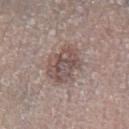Part of a total-body skin-imaging series; this lesion was reviewed on a skin check and was not flagged for biopsy. Located on the left lower leg. A 15 mm crop from a total-body photograph taken for skin-cancer surveillance. A male subject, roughly 55 years of age. Automated tile analysis of the lesion measured an outline eccentricity of about 0.75 (0 = round, 1 = elongated). The software also gave a lesion color around L≈50 a*≈15 b*≈20 in CIELAB and a lesion–skin lightness drop of about 10. And it measured a border-irregularity rating of about 2.5/10, a within-lesion color-variation index near 5.5/10, and peripheral color asymmetry of about 2. The software also gave lesion-presence confidence of about 85/100. Captured under white-light illumination. Measured at roughly 5 mm in maximum diameter.